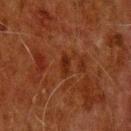Notes:
* biopsy status: catalogued during a skin exam; not biopsied
* body site: the head or neck
* image: 15 mm crop, total-body photography
* patient: male, aged 78–82